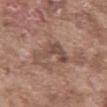Assessment:
Recorded during total-body skin imaging; not selected for excision or biopsy.
Background:
The patient is a male aged around 75. A region of skin cropped from a whole-body photographic capture, roughly 15 mm wide. The tile uses white-light illumination. From the abdomen.